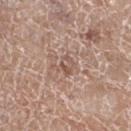Imaged during a routine full-body skin examination; the lesion was not biopsied and no histopathology is available. About 2.5 mm across. A female subject, aged 73 to 77. Automated image analysis of the tile measured an area of roughly 4 mm², a shape eccentricity near 0.6, and a symmetry-axis asymmetry near 0.45. It also reported an average lesion color of about L≈54 a*≈18 b*≈25 (CIELAB), roughly 8 lightness units darker than nearby skin, and a lesion-to-skin contrast of about 5.5 (normalized; higher = more distinct). It also reported a border-irregularity rating of about 5/10, a within-lesion color-variation index near 5.5/10, and a peripheral color-asymmetry measure near 2. A lesion tile, about 15 mm wide, cut from a 3D total-body photograph. From the left lower leg.